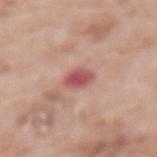biopsy_status: not biopsied; imaged during a skin examination
image:
  source: total-body photography crop
  field_of_view_mm: 15
lesion_size:
  long_diameter_mm_approx: 2.5
site: mid back
patient:
  sex: female
  age_approx: 75